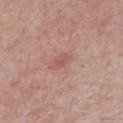workup — imaged on a skin check; not biopsied
diameter — about 2.5 mm
patient — male, aged approximately 55
location — the right upper arm
acquisition — total-body-photography crop, ~15 mm field of view
automated metrics — a mean CIELAB color near L≈55 a*≈21 b*≈27 and a lesion–skin lightness drop of about 7
lighting — white-light